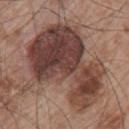Q: Was a biopsy performed?
A: no biopsy performed (imaged during a skin exam)
Q: Illumination type?
A: white-light illumination
Q: Lesion size?
A: ~10.5 mm (longest diameter)
Q: Lesion location?
A: the back
Q: Who is the patient?
A: male, aged 63 to 67
Q: How was this image acquired?
A: ~15 mm crop, total-body skin-cancer survey